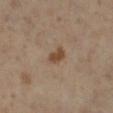follow-up: catalogued during a skin exam; not biopsied | lighting: cross-polarized illumination | image source: ~15 mm tile from a whole-body skin photo | TBP lesion metrics: roughly 10 lightness units darker than nearby skin and a normalized lesion–skin contrast near 8.5; peripheral color asymmetry of about 0.5 | subject: female, aged 38 to 42 | location: the left lower leg.